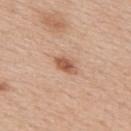A region of skin cropped from a whole-body photographic capture, roughly 15 mm wide. The lesion is located on the back. A male subject, approximately 60 years of age. About 3 mm across. Imaged with white-light lighting.